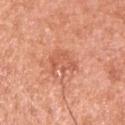Impression:
Imaged during a routine full-body skin examination; the lesion was not biopsied and no histopathology is available.
Acquisition and patient details:
Longest diameter approximately 4 mm. The lesion is on the left upper arm. A 15 mm close-up extracted from a 3D total-body photography capture. A male patient, aged 53 to 57.